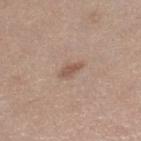Impression:
The lesion was tiled from a total-body skin photograph and was not biopsied.
Clinical summary:
From the right lower leg. Cropped from a whole-body photographic skin survey; the tile spans about 15 mm. A female patient, about 40 years old.